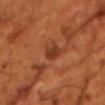Clinical impression: Imaged during a routine full-body skin examination; the lesion was not biopsied and no histopathology is available. Clinical summary: A 15 mm close-up tile from a total-body photography series done for melanoma screening. Located on the front of the torso. The tile uses cross-polarized illumination. Automated tile analysis of the lesion measured a lesion color around L≈34 a*≈25 b*≈31 in CIELAB, roughly 8 lightness units darker than nearby skin, and a lesion-to-skin contrast of about 7.5 (normalized; higher = more distinct). It also reported border irregularity of about 2.5 on a 0–10 scale and a color-variation rating of about 2.5/10. The analysis additionally found an automated nevus-likeness rating near 0 out of 100 and a detector confidence of about 100 out of 100 that the crop contains a lesion. Longest diameter approximately 2.5 mm. The subject is a male roughly 55 years of age.